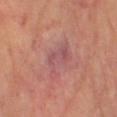Assessment:
Recorded during total-body skin imaging; not selected for excision or biopsy.
Clinical summary:
A female patient, aged around 65. Captured under cross-polarized illumination. The total-body-photography lesion software estimated a within-lesion color-variation index near 3.5/10 and peripheral color asymmetry of about 1. The software also gave a detector confidence of about 90 out of 100 that the crop contains a lesion. The lesion is on the left lower leg. A 15 mm close-up extracted from a 3D total-body photography capture. The lesion's longest dimension is about 4.5 mm.